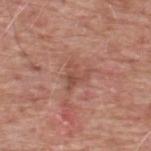The lesion was photographed on a routine skin check and not biopsied; there is no pathology result.
A 15 mm crop from a total-body photograph taken for skin-cancer surveillance.
A male subject, aged around 60.
This is a white-light tile.
On the back.
The recorded lesion diameter is about 3.5 mm.